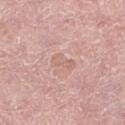Q: Was this lesion biopsied?
A: catalogued during a skin exam; not biopsied
Q: Lesion size?
A: ~3 mm (longest diameter)
Q: Lesion location?
A: the left lower leg
Q: What are the patient's age and sex?
A: female, aged 38–42
Q: How was this image acquired?
A: 15 mm crop, total-body photography